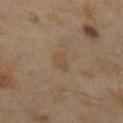<record>
  <biopsy_status>not biopsied; imaged during a skin examination</biopsy_status>
  <lighting>cross-polarized</lighting>
  <site>right lower leg</site>
  <image>
    <source>total-body photography crop</source>
    <field_of_view_mm>15</field_of_view_mm>
  </image>
  <lesion_size>
    <long_diameter_mm_approx>2.5</long_diameter_mm_approx>
  </lesion_size>
  <patient>
    <sex>female</sex>
    <age_approx>60</age_approx>
  </patient>
</record>